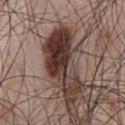{
  "biopsy_status": "not biopsied; imaged during a skin examination",
  "site": "chest",
  "patient": {
    "sex": "male",
    "age_approx": 50
  },
  "image": {
    "source": "total-body photography crop",
    "field_of_view_mm": 15
  },
  "lighting": "white-light"
}